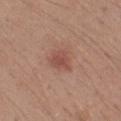A 15 mm crop from a total-body photograph taken for skin-cancer surveillance. From the left forearm. The patient is a male in their 20s. Captured under white-light illumination. About 2.5 mm across.